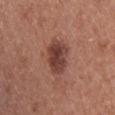Assessment:
The lesion was photographed on a routine skin check and not biopsied; there is no pathology result.
Acquisition and patient details:
The lesion's longest dimension is about 4 mm. The tile uses white-light illumination. From the back. A female patient aged 38 to 42. A 15 mm crop from a total-body photograph taken for skin-cancer surveillance.